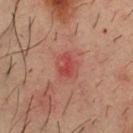Assessment: Recorded during total-body skin imaging; not selected for excision or biopsy. Clinical summary: A male subject in their mid-30s. Measured at roughly 3.5 mm in maximum diameter. Automated tile analysis of the lesion measured peripheral color asymmetry of about 2. And it measured a classifier nevus-likeness of about 0/100 and lesion-presence confidence of about 100/100. On the chest. A roughly 15 mm field-of-view crop from a total-body skin photograph. Captured under cross-polarized illumination.